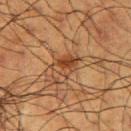<record>
<biopsy_status>not biopsied; imaged during a skin examination</biopsy_status>
<image>
  <source>total-body photography crop</source>
  <field_of_view_mm>15</field_of_view_mm>
</image>
<site>right upper arm</site>
<lighting>cross-polarized</lighting>
<patient>
  <sex>male</sex>
  <age_approx>60</age_approx>
</patient>
</record>